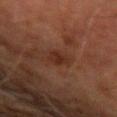  biopsy_status: not biopsied; imaged during a skin examination
  lesion_size:
    long_diameter_mm_approx: 3.0
  lighting: cross-polarized
  image:
    source: total-body photography crop
    field_of_view_mm: 15
  patient:
    sex: male
    age_approx: 70
  site: right upper arm